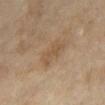The lesion was tiled from a total-body skin photograph and was not biopsied.
An algorithmic analysis of the crop reported roughly 6 lightness units darker than nearby skin. The analysis additionally found a nevus-likeness score of about 0/100 and a lesion-detection confidence of about 100/100.
This image is a 15 mm lesion crop taken from a total-body photograph.
On the right thigh.
A female patient, roughly 50 years of age.
Imaged with cross-polarized lighting.